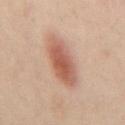Assessment: Part of a total-body skin-imaging series; this lesion was reviewed on a skin check and was not flagged for biopsy. Image and clinical context: The tile uses cross-polarized illumination. Approximately 5.5 mm at its widest. A male subject, aged approximately 45. A region of skin cropped from a whole-body photographic capture, roughly 15 mm wide. The lesion is on the back. The lesion-visualizer software estimated an eccentricity of roughly 0.8 and a symmetry-axis asymmetry near 0.15. And it measured a within-lesion color-variation index near 5/10. It also reported a classifier nevus-likeness of about 100/100.